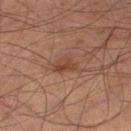The lesion was photographed on a routine skin check and not biopsied; there is no pathology result.
Approximately 3 mm at its widest.
Cropped from a total-body skin-imaging series; the visible field is about 15 mm.
The tile uses cross-polarized illumination.
From the left lower leg.
A male patient, roughly 55 years of age.